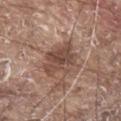| field | value |
|---|---|
| patient | male, aged around 80 |
| imaging modality | ~15 mm tile from a whole-body skin photo |
| anatomic site | the upper back |